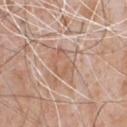Acquisition and patient details:
A 15 mm close-up extracted from a 3D total-body photography capture. About 4 mm across. The patient is a male roughly 65 years of age. Imaged with white-light lighting. The lesion is located on the chest. Automated image analysis of the tile measured a footprint of about 10 mm², an outline eccentricity of about 0.6 (0 = round, 1 = elongated), and a shape-asymmetry score of about 0.2 (0 = symmetric).